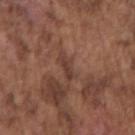{"biopsy_status": "not biopsied; imaged during a skin examination", "lesion_size": {"long_diameter_mm_approx": 2.5}, "patient": {"sex": "male", "age_approx": 75}, "site": "right upper arm", "lighting": "white-light", "automated_metrics": {"area_mm2_approx": 3.0, "eccentricity": 0.85, "shape_asymmetry": 0.3, "vs_skin_darker_L": 7.0, "border_irregularity_0_10": 3.5, "color_variation_0_10": 0.0, "peripheral_color_asymmetry": 0.0, "nevus_likeness_0_100": 0, "lesion_detection_confidence_0_100": 50}, "image": {"source": "total-body photography crop", "field_of_view_mm": 15}}